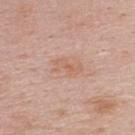Impression:
This lesion was catalogued during total-body skin photography and was not selected for biopsy.
Clinical summary:
The lesion is on the upper back. The recorded lesion diameter is about 3.5 mm. Automated tile analysis of the lesion measured a lesion area of about 4 mm², an eccentricity of roughly 0.9, and two-axis asymmetry of about 0.5. It also reported a classifier nevus-likeness of about 0/100 and a detector confidence of about 100 out of 100 that the crop contains a lesion. This image is a 15 mm lesion crop taken from a total-body photograph. The subject is a male aged 38 to 42. The tile uses white-light illumination.